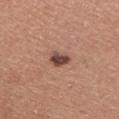Captured during whole-body skin photography for melanoma surveillance; the lesion was not biopsied. Measured at roughly 2.5 mm in maximum diameter. This image is a 15 mm lesion crop taken from a total-body photograph. A male subject approximately 45 years of age. The lesion is located on the chest.